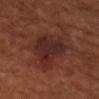Diagnosis:
On excision, pathology confirmed an actinic keratosis — an indeterminate (borderline) lesion.